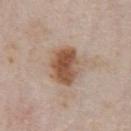Clinical impression:
Captured during whole-body skin photography for melanoma surveillance; the lesion was not biopsied.
Image and clinical context:
Measured at roughly 5 mm in maximum diameter. This image is a 15 mm lesion crop taken from a total-body photograph. Captured under white-light illumination. A male subject about 75 years old. Located on the chest. An algorithmic analysis of the crop reported a lesion color around L≈52 a*≈19 b*≈30 in CIELAB, about 14 CIELAB-L* units darker than the surrounding skin, and a lesion-to-skin contrast of about 10 (normalized; higher = more distinct). And it measured a classifier nevus-likeness of about 100/100.